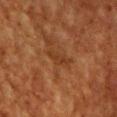Assessment: Part of a total-body skin-imaging series; this lesion was reviewed on a skin check and was not flagged for biopsy. Image and clinical context: Cropped from a total-body skin-imaging series; the visible field is about 15 mm. The subject is a male aged 58–62. The tile uses cross-polarized illumination. The lesion's longest dimension is about 3 mm. On the front of the torso. The total-body-photography lesion software estimated a mean CIELAB color near L≈30 a*≈20 b*≈31, a lesion–skin lightness drop of about 5, and a normalized lesion–skin contrast near 5.5. The analysis additionally found a border-irregularity index near 5/10, a color-variation rating of about 0/10, and radial color variation of about 0.